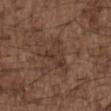notes = no biopsy performed (imaged during a skin exam) | lighting = white-light illumination | diameter = ~5 mm (longest diameter) | patient = male, aged 48 to 52 | image = ~15 mm tile from a whole-body skin photo | body site = the upper back.